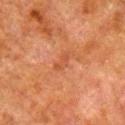Findings:
- notes: catalogued during a skin exam; not biopsied
- acquisition: total-body-photography crop, ~15 mm field of view
- tile lighting: cross-polarized illumination
- patient: male, roughly 80 years of age
- site: the right lower leg
- TBP lesion metrics: a lesion color around L≈41 a*≈25 b*≈32 in CIELAB and a normalized lesion–skin contrast near 5; a border-irregularity rating of about 3.5/10, a within-lesion color-variation index near 0/10, and a peripheral color-asymmetry measure near 0; lesion-presence confidence of about 100/100